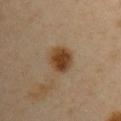biopsy status: no biopsy performed (imaged during a skin exam)
TBP lesion metrics: a footprint of about 9 mm² and an outline eccentricity of about 0.45 (0 = round, 1 = elongated); a border-irregularity rating of about 1.5/10, a within-lesion color-variation index near 4.5/10, and peripheral color asymmetry of about 1; a nevus-likeness score of about 100/100 and a detector confidence of about 100 out of 100 that the crop contains a lesion
subject: female, aged 43 to 47
location: the arm
acquisition: 15 mm crop, total-body photography
tile lighting: cross-polarized illumination
diameter: about 3.5 mm